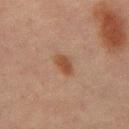Case summary:
– follow-up: catalogued during a skin exam; not biopsied
– anatomic site: the front of the torso
– subject: male, aged 63 to 67
– size: ~3 mm (longest diameter)
– automated lesion analysis: about 8 CIELAB-L* units darker than the surrounding skin and a lesion-to-skin contrast of about 8 (normalized; higher = more distinct); a border-irregularity index near 2/10, a within-lesion color-variation index near 2/10, and peripheral color asymmetry of about 0.5
– illumination: cross-polarized illumination
– acquisition: ~15 mm crop, total-body skin-cancer survey